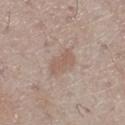Clinical impression: This lesion was catalogued during total-body skin photography and was not selected for biopsy. Image and clinical context: Automated image analysis of the tile measured an area of roughly 4.5 mm², a shape eccentricity near 0.9, and a symmetry-axis asymmetry near 0.3. The analysis additionally found a classifier nevus-likeness of about 20/100. On the left lower leg. Measured at roughly 3.5 mm in maximum diameter. A region of skin cropped from a whole-body photographic capture, roughly 15 mm wide. Captured under white-light illumination. A male patient aged 68 to 72.